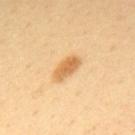<case>
<image>
  <source>total-body photography crop</source>
  <field_of_view_mm>15</field_of_view_mm>
</image>
<lesion_size>
  <long_diameter_mm_approx>3.5</long_diameter_mm_approx>
</lesion_size>
<automated_metrics>
  <cielab_L>67</cielab_L>
  <cielab_a>21</cielab_a>
  <cielab_b>45</cielab_b>
  <vs_skin_darker_L>12.0</vs_skin_darker_L>
  <vs_skin_contrast_norm>8.0</vs_skin_contrast_norm>
  <border_irregularity_0_10>2.5</border_irregularity_0_10>
  <color_variation_0_10>3.0</color_variation_0_10>
  <nevus_likeness_0_100>95</nevus_likeness_0_100>
  <lesion_detection_confidence_0_100>100</lesion_detection_confidence_0_100>
</automated_metrics>
<site>upper back</site>
<patient>
  <sex>male</sex>
  <age_approx>40</age_approx>
</patient>
<lighting>cross-polarized</lighting>
</case>